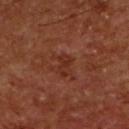| feature | finding |
|---|---|
| notes | imaged on a skin check; not biopsied |
| image-analysis metrics | a lesion area of about 3 mm² and a shape eccentricity near 0.85; lesion-presence confidence of about 100/100 |
| acquisition | ~15 mm crop, total-body skin-cancer survey |
| lighting | cross-polarized illumination |
| lesion size | ≈2.5 mm |
| subject | male, aged approximately 65 |
| location | the upper back |